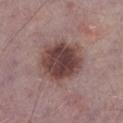Q: Was a biopsy performed?
A: catalogued during a skin exam; not biopsied
Q: Patient demographics?
A: male, in their mid-70s
Q: How was this image acquired?
A: 15 mm crop, total-body photography
Q: Where on the body is the lesion?
A: the left lower leg